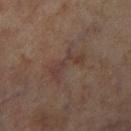Q: Was a biopsy performed?
A: no biopsy performed (imaged during a skin exam)
Q: How was the tile lit?
A: cross-polarized
Q: What kind of image is this?
A: ~15 mm tile from a whole-body skin photo
Q: What is the lesion's diameter?
A: ≈5 mm
Q: Who is the patient?
A: male, about 70 years old
Q: What is the anatomic site?
A: the right lower leg